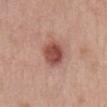workup: no biopsy performed (imaged during a skin exam); size: ~3.5 mm (longest diameter); site: the abdomen; image source: ~15 mm tile from a whole-body skin photo; patient: female, roughly 40 years of age; illumination: white-light illumination.